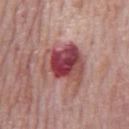Notes:
• workup — catalogued during a skin exam; not biopsied
• lighting — white-light
• image source — total-body-photography crop, ~15 mm field of view
• body site — the mid back
• lesion diameter — about 5.5 mm
• subject — male, about 75 years old